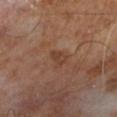Impression:
This lesion was catalogued during total-body skin photography and was not selected for biopsy.
Context:
A close-up tile cropped from a whole-body skin photograph, about 15 mm across. The total-body-photography lesion software estimated an average lesion color of about L≈39 a*≈19 b*≈28 (CIELAB), roughly 6 lightness units darker than nearby skin, and a normalized border contrast of about 5.5. And it measured a border-irregularity rating of about 3.5/10, internal color variation of about 2 on a 0–10 scale, and a peripheral color-asymmetry measure near 0.5. It also reported a classifier nevus-likeness of about 0/100 and a lesion-detection confidence of about 100/100. This is a cross-polarized tile. A male patient about 65 years old.